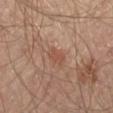biopsy status: total-body-photography surveillance lesion; no biopsy
tile lighting: cross-polarized
patient: male, roughly 65 years of age
diameter: about 3 mm
automated lesion analysis: a lesion area of about 4.5 mm², a shape eccentricity near 0.85, and a shape-asymmetry score of about 0.3 (0 = symmetric); a lesion-to-skin contrast of about 5.5 (normalized; higher = more distinct)
body site: the left thigh
image: ~15 mm crop, total-body skin-cancer survey